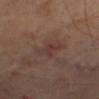Impression: Recorded during total-body skin imaging; not selected for excision or biopsy. Image and clinical context: The subject is a male aged 68–72. Automated image analysis of the tile measured radial color variation of about 1.5. The analysis additionally found a nevus-likeness score of about 0/100. From the right thigh. A 15 mm crop from a total-body photograph taken for skin-cancer surveillance.